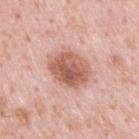Recorded during total-body skin imaging; not selected for excision or biopsy. A 15 mm crop from a total-body photograph taken for skin-cancer surveillance. From the upper back. A male subject, about 35 years old. This is a white-light tile.